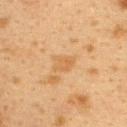| field | value |
|---|---|
| follow-up | imaged on a skin check; not biopsied |
| automated metrics | a border-irregularity rating of about 3.5/10 and a peripheral color-asymmetry measure near 1; a detector confidence of about 100 out of 100 that the crop contains a lesion |
| body site | the upper back |
| acquisition | ~15 mm tile from a whole-body skin photo |
| subject | female, roughly 40 years of age |
| lighting | cross-polarized |
| size | ~2.5 mm (longest diameter) |